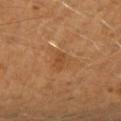follow-up: imaged on a skin check; not biopsied
image-analysis metrics: a mean CIELAB color near L≈47 a*≈23 b*≈38 and roughly 7 lightness units darker than nearby skin
imaging modality: ~15 mm tile from a whole-body skin photo
location: the right forearm
subject: male, approximately 40 years of age
lighting: cross-polarized